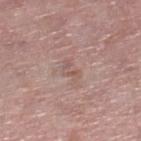workup — no biopsy performed (imaged during a skin exam)
subject — female, approximately 60 years of age
TBP lesion metrics — a footprint of about 3 mm², a shape eccentricity near 0.85, and a symmetry-axis asymmetry near 0.55; a lesion color around L≈55 a*≈19 b*≈23 in CIELAB and a normalized border contrast of about 4.5; border irregularity of about 6.5 on a 0–10 scale, a color-variation rating of about 0/10, and a peripheral color-asymmetry measure near 0; a lesion-detection confidence of about 100/100
diameter — about 3 mm
site — the right lower leg
acquisition — total-body-photography crop, ~15 mm field of view